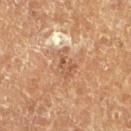The lesion was tiled from a total-body skin photograph and was not biopsied. The subject is a male about 75 years old. A roughly 15 mm field-of-view crop from a total-body skin photograph. The lesion's longest dimension is about 3.5 mm. The lesion is on the left lower leg. This is a cross-polarized tile.